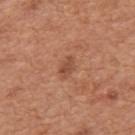The lesion was photographed on a routine skin check and not biopsied; there is no pathology result. The subject is a male aged 63 to 67. The lesion is located on the mid back. Automated image analysis of the tile measured border irregularity of about 2.5 on a 0–10 scale and a color-variation rating of about 1/10. Measured at roughly 2.5 mm in maximum diameter. A 15 mm crop from a total-body photograph taken for skin-cancer surveillance.